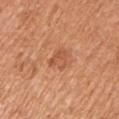Findings:
– tile lighting · white-light illumination
– site · the arm
– image source · total-body-photography crop, ~15 mm field of view
– patient · male, aged around 55
– size · about 3 mm
– TBP lesion metrics · an area of roughly 5 mm² and a shape-asymmetry score of about 0.4 (0 = symmetric); a lesion–skin lightness drop of about 8 and a normalized lesion–skin contrast near 5.5